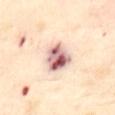• workup · imaged on a skin check; not biopsied
• location · the abdomen
• automated lesion analysis · a mean CIELAB color near L≈69 a*≈22 b*≈23, a lesion–skin lightness drop of about 21, and a normalized border contrast of about 12; a classifier nevus-likeness of about 0/100 and a lesion-detection confidence of about 100/100
• lesion diameter · about 4 mm
• illumination · cross-polarized
• subject · female, aged around 55
• image · 15 mm crop, total-body photography